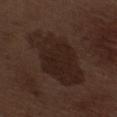follow-up — no biopsy performed (imaged during a skin exam)
size — about 9 mm
patient — male, about 70 years old
location — the leg
image source — ~15 mm tile from a whole-body skin photo
automated lesion analysis — an area of roughly 31 mm² and a shape-asymmetry score of about 0.2 (0 = symmetric); a lesion color around L≈21 a*≈14 b*≈19 in CIELAB and a lesion-to-skin contrast of about 8 (normalized; higher = more distinct); border irregularity of about 3 on a 0–10 scale, internal color variation of about 2.5 on a 0–10 scale, and a peripheral color-asymmetry measure near 1; a classifier nevus-likeness of about 0/100 and a detector confidence of about 100 out of 100 that the crop contains a lesion
tile lighting — white-light